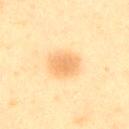biopsy status: imaged on a skin check; not biopsied
image: ~15 mm crop, total-body skin-cancer survey
location: the right upper arm
subject: male, in their mid- to late 40s